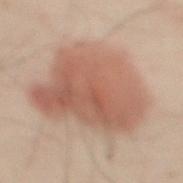Impression: Captured during whole-body skin photography for melanoma surveillance; the lesion was not biopsied. Context: Imaged with cross-polarized lighting. Cropped from a total-body skin-imaging series; the visible field is about 15 mm. The lesion is on the abdomen. A male subject approximately 50 years of age. The lesion-visualizer software estimated an area of roughly 55 mm², an eccentricity of roughly 0.6, and a shape-asymmetry score of about 0.25 (0 = symmetric). It also reported an average lesion color of about L≈46 a*≈17 b*≈23 (CIELAB), a lesion–skin lightness drop of about 9, and a lesion-to-skin contrast of about 7 (normalized; higher = more distinct). It also reported a nevus-likeness score of about 100/100 and a detector confidence of about 100 out of 100 that the crop contains a lesion.